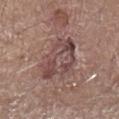{"image": {"source": "total-body photography crop", "field_of_view_mm": 15}, "site": "leg", "lesion_size": {"long_diameter_mm_approx": 6.0}, "lighting": "white-light", "automated_metrics": {"area_mm2_approx": 16.0, "eccentricity": 0.8, "shape_asymmetry": 0.25, "border_irregularity_0_10": 3.5, "peripheral_color_asymmetry": 2.0}, "patient": {"sex": "male", "age_approx": 65}}